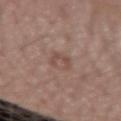Imaged during a routine full-body skin examination; the lesion was not biopsied and no histopathology is available.
The lesion is on the left forearm.
Imaged with white-light lighting.
A lesion tile, about 15 mm wide, cut from a 3D total-body photograph.
A female subject, approximately 55 years of age.
The total-body-photography lesion software estimated a lesion–skin lightness drop of about 7 and a lesion-to-skin contrast of about 5.5 (normalized; higher = more distinct).